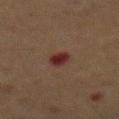{"biopsy_status": "not biopsied; imaged during a skin examination", "automated_metrics": {"area_mm2_approx": 4.0, "eccentricity": 0.65, "shape_asymmetry": 0.15, "nevus_likeness_0_100": 0, "lesion_detection_confidence_0_100": 100}, "patient": {"sex": "female", "age_approx": 50}, "site": "abdomen", "lighting": "cross-polarized", "lesion_size": {"long_diameter_mm_approx": 2.5}, "image": {"source": "total-body photography crop", "field_of_view_mm": 15}}